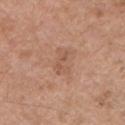This lesion was catalogued during total-body skin photography and was not selected for biopsy.
Approximately 3.5 mm at its widest.
A male subject aged around 55.
The lesion is on the chest.
Imaged with white-light lighting.
Cropped from a total-body skin-imaging series; the visible field is about 15 mm.